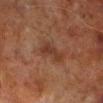No biopsy was performed on this lesion — it was imaged during a full skin examination and was not determined to be concerning. The total-body-photography lesion software estimated a border-irregularity index near 4.5/10 and a peripheral color-asymmetry measure near 0.5. The software also gave a nevus-likeness score of about 0/100 and lesion-presence confidence of about 100/100. Captured under cross-polarized illumination. The patient is a male roughly 70 years of age. This image is a 15 mm lesion crop taken from a total-body photograph. From the right lower leg.